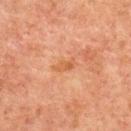<tbp_lesion>
  <biopsy_status>not biopsied; imaged during a skin examination</biopsy_status>
  <lighting>cross-polarized</lighting>
  <image>
    <source>total-body photography crop</source>
    <field_of_view_mm>15</field_of_view_mm>
  </image>
  <automated_metrics>
    <border_irregularity_0_10>4.0</border_irregularity_0_10>
    <color_variation_0_10>0.0</color_variation_0_10>
    <peripheral_color_asymmetry>0.0</peripheral_color_asymmetry>
    <nevus_likeness_0_100>0</nevus_likeness_0_100>
    <lesion_detection_confidence_0_100>100</lesion_detection_confidence_0_100>
  </automated_metrics>
  <site>upper back</site>
  <patient>
    <sex>male</sex>
    <age_approx>60</age_approx>
  </patient>
</tbp_lesion>